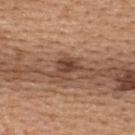Q: Was a biopsy performed?
A: imaged on a skin check; not biopsied